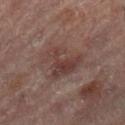| field | value |
|---|---|
| site | the left leg |
| illumination | cross-polarized |
| acquisition | total-body-photography crop, ~15 mm field of view |
| subject | female, aged approximately 80 |